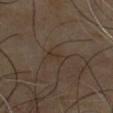follow-up: no biopsy performed (imaged during a skin exam); body site: the chest; subject: male, aged 63–67; size: about 3 mm; lighting: cross-polarized illumination; acquisition: ~15 mm tile from a whole-body skin photo.